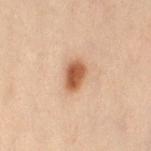<record>
<biopsy_status>not biopsied; imaged during a skin examination</biopsy_status>
<image>
  <source>total-body photography crop</source>
  <field_of_view_mm>15</field_of_view_mm>
</image>
<patient>
  <sex>female</sex>
  <age_approx>55</age_approx>
</patient>
<lesion_size>
  <long_diameter_mm_approx>3.5</long_diameter_mm_approx>
</lesion_size>
<site>abdomen</site>
<lighting>cross-polarized</lighting>
</record>